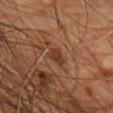biopsy_status: not biopsied; imaged during a skin examination
lighting: cross-polarized
site: front of the torso
automated_metrics:
  area_mm2_approx: 3.5
  eccentricity: 0.8
  shape_asymmetry: 0.3
patient:
  sex: male
  age_approx: 85+
lesion_size:
  long_diameter_mm_approx: 2.5
image:
  source: total-body photography crop
  field_of_view_mm: 15